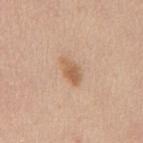Case summary:
– follow-up — no biopsy performed (imaged during a skin exam)
– anatomic site — the mid back
– subject — female, aged approximately 55
– image source — 15 mm crop, total-body photography